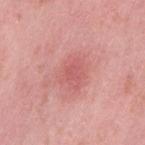subject: female, aged 38–42
imaging modality: ~15 mm tile from a whole-body skin photo
location: the left thigh